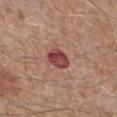follow-up — imaged on a skin check; not biopsied | tile lighting — white-light illumination | image — total-body-photography crop, ~15 mm field of view | subject — male, aged around 65 | body site — the right lower leg.